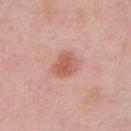Case summary:
- workup · total-body-photography surveillance lesion; no biopsy
- automated metrics · a lesion area of about 7.5 mm², an outline eccentricity of about 0.6 (0 = round, 1 = elongated), and a shape-asymmetry score of about 0.15 (0 = symmetric); a lesion–skin lightness drop of about 10 and a lesion-to-skin contrast of about 7 (normalized; higher = more distinct)
- patient · male, aged 58 to 62
- lesion size · ~3.5 mm (longest diameter)
- imaging modality · total-body-photography crop, ~15 mm field of view
- body site · the chest